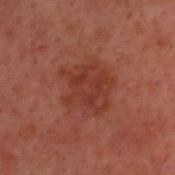Impression:
The lesion was photographed on a routine skin check and not biopsied; there is no pathology result.
Context:
The lesion is on the head or neck. This is a cross-polarized tile. The recorded lesion diameter is about 6 mm. The lesion-visualizer software estimated an average lesion color of about L≈28 a*≈22 b*≈23 (CIELAB), a lesion–skin lightness drop of about 5, and a normalized border contrast of about 5.5. The patient is a male about 50 years old. A region of skin cropped from a whole-body photographic capture, roughly 15 mm wide.